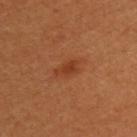Assessment:
Part of a total-body skin-imaging series; this lesion was reviewed on a skin check and was not flagged for biopsy.
Context:
An algorithmic analysis of the crop reported a mean CIELAB color near L≈39 a*≈28 b*≈37, a lesion–skin lightness drop of about 8, and a normalized border contrast of about 7. It also reported a classifier nevus-likeness of about 95/100 and a lesion-detection confidence of about 100/100. Cropped from a whole-body photographic skin survey; the tile spans about 15 mm. The subject is a female aged around 50. Measured at roughly 2.5 mm in maximum diameter.